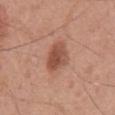workup: total-body-photography surveillance lesion; no biopsy
size: ≈4 mm
subject: male, aged around 55
site: the front of the torso
image: ~15 mm crop, total-body skin-cancer survey
tile lighting: white-light illumination